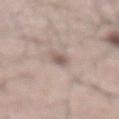A roughly 15 mm field-of-view crop from a total-body skin photograph.
The lesion is located on the abdomen.
A male patient about 65 years old.